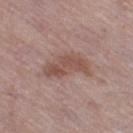Impression:
Imaged during a routine full-body skin examination; the lesion was not biopsied and no histopathology is available.
Clinical summary:
Measured at roughly 5.5 mm in maximum diameter. Cropped from a total-body skin-imaging series; the visible field is about 15 mm. The subject is a female about 60 years old. Automated tile analysis of the lesion measured an area of roughly 14 mm² and two-axis asymmetry of about 0.4. It also reported an average lesion color of about L≈52 a*≈19 b*≈24 (CIELAB). And it measured an automated nevus-likeness rating near 0 out of 100 and a lesion-detection confidence of about 100/100. Captured under white-light illumination. The lesion is located on the right thigh.